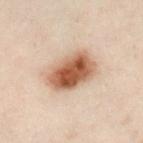* follow-up · total-body-photography surveillance lesion; no biopsy
* diameter · ~6 mm (longest diameter)
* illumination · cross-polarized illumination
* subject · male, aged 48 to 52
* site · the left leg
* image-analysis metrics · an outline eccentricity of about 0.8 (0 = round, 1 = elongated); border irregularity of about 1.5 on a 0–10 scale, a color-variation rating of about 6/10, and radial color variation of about 2
* imaging modality · ~15 mm crop, total-body skin-cancer survey